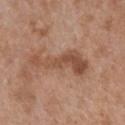Q: Was a biopsy performed?
A: total-body-photography surveillance lesion; no biopsy